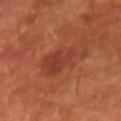Recorded during total-body skin imaging; not selected for excision or biopsy. Automated tile analysis of the lesion measured a lesion area of about 11 mm², an eccentricity of roughly 0.7, and a symmetry-axis asymmetry near 0.45. The analysis additionally found roughly 6 lightness units darker than nearby skin and a lesion-to-skin contrast of about 5.5 (normalized; higher = more distinct). The analysis additionally found radial color variation of about 1. It also reported an automated nevus-likeness rating near 5 out of 100. Located on the right forearm. The subject is a male roughly 60 years of age. Cropped from a whole-body photographic skin survey; the tile spans about 15 mm.